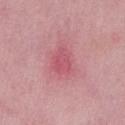The lesion was photographed on a routine skin check and not biopsied; there is no pathology result. Cropped from a whole-body photographic skin survey; the tile spans about 15 mm. Imaged with white-light lighting. The lesion is on the mid back. A male subject, about 50 years old. The lesion's longest dimension is about 3 mm.